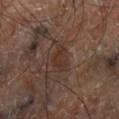- notes — catalogued during a skin exam; not biopsied
- lesion size — about 3 mm
- illumination — cross-polarized
- site — the right lower leg
- acquisition — 15 mm crop, total-body photography
- image-analysis metrics — a nevus-likeness score of about 0/100 and lesion-presence confidence of about 95/100